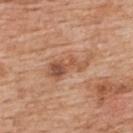{"lighting": "white-light", "image": {"source": "total-body photography crop", "field_of_view_mm": 15}, "patient": {"sex": "male", "age_approx": 60}, "automated_metrics": {"eccentricity": 0.9, "vs_skin_darker_L": 9.0, "vs_skin_contrast_norm": 6.5, "border_irregularity_0_10": 5.5, "color_variation_0_10": 6.0, "peripheral_color_asymmetry": 2.0}, "site": "upper back", "lesion_size": {"long_diameter_mm_approx": 6.0}}